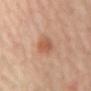<case>
  <biopsy_status>not biopsied; imaged during a skin examination</biopsy_status>
  <automated_metrics>
    <border_irregularity_0_10>1.5</border_irregularity_0_10>
    <color_variation_0_10>2.0</color_variation_0_10>
    <peripheral_color_asymmetry>0.5</peripheral_color_asymmetry>
  </automated_metrics>
  <lighting>cross-polarized</lighting>
  <lesion_size>
    <long_diameter_mm_approx>3.0</long_diameter_mm_approx>
  </lesion_size>
  <patient>
    <sex>male</sex>
    <age_approx>70</age_approx>
  </patient>
  <image>
    <source>total-body photography crop</source>
    <field_of_view_mm>15</field_of_view_mm>
  </image>
  <site>back</site>
</case>